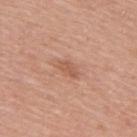No biopsy was performed on this lesion — it was imaged during a full skin examination and was not determined to be concerning.
Automated tile analysis of the lesion measured a normalized border contrast of about 5.5.
Cropped from a whole-body photographic skin survey; the tile spans about 15 mm.
A female subject, roughly 60 years of age.
The lesion is on the upper back.
The recorded lesion diameter is about 3 mm.
Imaged with white-light lighting.